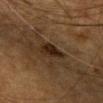- workup · no biopsy performed (imaged during a skin exam)
- image · 15 mm crop, total-body photography
- anatomic site · the head or neck
- lighting · cross-polarized illumination
- diameter · ≈3.5 mm
- automated metrics · a lesion area of about 6 mm², an outline eccentricity of about 0.7 (0 = round, 1 = elongated), and two-axis asymmetry of about 0.3; border irregularity of about 2.5 on a 0–10 scale, a color-variation rating of about 3.5/10, and a peripheral color-asymmetry measure near 1; a nevus-likeness score of about 70/100 and a detector confidence of about 100 out of 100 that the crop contains a lesion
- subject · female, aged around 55